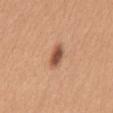No biopsy was performed on this lesion — it was imaged during a full skin examination and was not determined to be concerning.